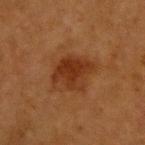Clinical impression: Captured during whole-body skin photography for melanoma surveillance; the lesion was not biopsied. Acquisition and patient details: A female patient, approximately 40 years of age. A region of skin cropped from a whole-body photographic capture, roughly 15 mm wide. The lesion is located on the upper back.